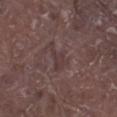workup=total-body-photography surveillance lesion; no biopsy
site=the right lower leg
patient=male, aged 78–82
size=~4 mm (longest diameter)
imaging modality=total-body-photography crop, ~15 mm field of view
lighting=white-light